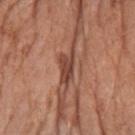Findings:
* biopsy status — no biopsy performed (imaged during a skin exam)
* imaging modality — 15 mm crop, total-body photography
* diameter — ~4 mm (longest diameter)
* body site — the right forearm
* patient — female, aged around 75
* illumination — white-light